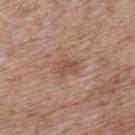<tbp_lesion>
<biopsy_status>not biopsied; imaged during a skin examination</biopsy_status>
<image>
  <source>total-body photography crop</source>
  <field_of_view_mm>15</field_of_view_mm>
</image>
<patient>
  <sex>male</sex>
  <age_approx>70</age_approx>
</patient>
<site>mid back</site>
</tbp_lesion>